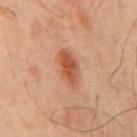Q: Is there a histopathology result?
A: imaged on a skin check; not biopsied
Q: Patient demographics?
A: male, aged 63–67
Q: Automated lesion metrics?
A: an average lesion color of about L≈52 a*≈26 b*≈34 (CIELAB), a lesion–skin lightness drop of about 11, and a normalized border contrast of about 8; a border-irregularity rating of about 2.5/10, a within-lesion color-variation index near 4.5/10, and peripheral color asymmetry of about 1.5; an automated nevus-likeness rating near 90 out of 100 and lesion-presence confidence of about 100/100
Q: What is the anatomic site?
A: the mid back
Q: Lesion size?
A: ≈4.5 mm
Q: What kind of image is this?
A: ~15 mm crop, total-body skin-cancer survey